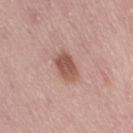Part of a total-body skin-imaging series; this lesion was reviewed on a skin check and was not flagged for biopsy. A 15 mm close-up tile from a total-body photography series done for melanoma screening. Approximately 4 mm at its widest. Imaged with white-light lighting. A female subject roughly 50 years of age. The lesion is on the right thigh. Automated image analysis of the tile measured radial color variation of about 1.5. The software also gave a nevus-likeness score of about 85/100 and a detector confidence of about 100 out of 100 that the crop contains a lesion.